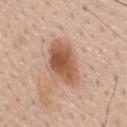A roughly 15 mm field-of-view crop from a total-body skin photograph.
The subject is a male roughly 60 years of age.
On the upper back.
The recorded lesion diameter is about 5.5 mm.